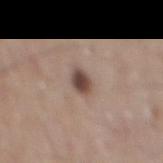Impression:
The lesion was tiled from a total-body skin photograph and was not biopsied.
Context:
A 15 mm crop from a total-body photograph taken for skin-cancer surveillance. On the mid back. Automated image analysis of the tile measured a lesion color around L≈46 a*≈16 b*≈22 in CIELAB, roughly 14 lightness units darker than nearby skin, and a normalized lesion–skin contrast near 10.5. The patient is a male aged 73–77. Imaged with white-light lighting. The lesion's longest dimension is about 2.5 mm.